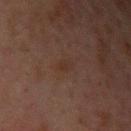<tbp_lesion>
  <site>left upper arm</site>
  <image>
    <source>total-body photography crop</source>
    <field_of_view_mm>15</field_of_view_mm>
  </image>
  <automated_metrics>
    <border_irregularity_0_10>3.5</border_irregularity_0_10>
    <color_variation_0_10>0.0</color_variation_0_10>
    <peripheral_color_asymmetry>0.0</peripheral_color_asymmetry>
  </automated_metrics>
  <patient>
    <sex>male</sex>
    <age_approx>30</age_approx>
  </patient>
  <lesion_size>
    <long_diameter_mm_approx>2.5</long_diameter_mm_approx>
  </lesion_size>
  <lighting>cross-polarized</lighting>
</tbp_lesion>